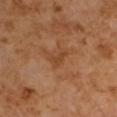Recorded during total-body skin imaging; not selected for excision or biopsy. An algorithmic analysis of the crop reported an outline eccentricity of about 0.85 (0 = round, 1 = elongated). It also reported a lesion color around L≈42 a*≈23 b*≈35 in CIELAB, roughly 6 lightness units darker than nearby skin, and a normalized border contrast of about 5.5. The software also gave a color-variation rating of about 0/10 and a peripheral color-asymmetry measure near 0. Captured under cross-polarized illumination. A 15 mm crop from a total-body photograph taken for skin-cancer surveillance. The subject is a male approximately 65 years of age.